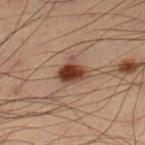The lesion was photographed on a routine skin check and not biopsied; there is no pathology result.
The tile uses cross-polarized illumination.
The lesion is located on the left lower leg.
A lesion tile, about 15 mm wide, cut from a 3D total-body photograph.
A male patient aged around 55.
Automated image analysis of the tile measured a mean CIELAB color near L≈33 a*≈18 b*≈23.
Longest diameter approximately 3 mm.